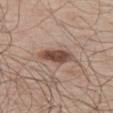follow-up: no biopsy performed (imaged during a skin exam) | illumination: white-light | body site: the left thigh | patient: male, aged approximately 60 | image source: ~15 mm tile from a whole-body skin photo | automated lesion analysis: a footprint of about 7.5 mm² and an eccentricity of roughly 0.9; a mean CIELAB color near L≈47 a*≈18 b*≈25, about 14 CIELAB-L* units darker than the surrounding skin, and a normalized border contrast of about 10.5; a within-lesion color-variation index near 3.5/10 and peripheral color asymmetry of about 1; an automated nevus-likeness rating near 95 out of 100 and lesion-presence confidence of about 100/100 | diameter: about 4.5 mm.